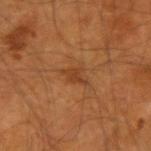Clinical impression:
The lesion was photographed on a routine skin check and not biopsied; there is no pathology result.
Clinical summary:
The patient is a male aged 53 to 57. A lesion tile, about 15 mm wide, cut from a 3D total-body photograph. The lesion is on the right forearm.